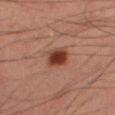The lesion was tiled from a total-body skin photograph and was not biopsied. The patient is a male roughly 60 years of age. Located on the leg. About 2.5 mm across. A 15 mm crop from a total-body photograph taken for skin-cancer surveillance.